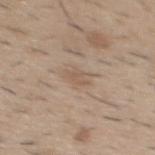Case summary:
* notes — catalogued during a skin exam; not biopsied